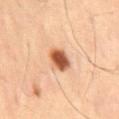The lesion was photographed on a routine skin check and not biopsied; there is no pathology result. A 15 mm crop from a total-body photograph taken for skin-cancer surveillance. On the mid back. Longest diameter approximately 3.5 mm. The subject is a male aged approximately 55. Captured under cross-polarized illumination.